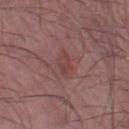{
  "biopsy_status": "not biopsied; imaged during a skin examination",
  "lesion_size": {
    "long_diameter_mm_approx": 2.5
  },
  "image": {
    "source": "total-body photography crop",
    "field_of_view_mm": 15
  },
  "site": "left thigh",
  "patient": {
    "sex": "male",
    "age_approx": 50
  },
  "automated_metrics": {
    "cielab_L": 42,
    "cielab_a": 24,
    "cielab_b": 20,
    "vs_skin_darker_L": 6.0,
    "vs_skin_contrast_norm": 5.5
  },
  "lighting": "white-light"
}